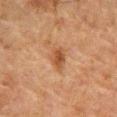Q: Is there a histopathology result?
A: imaged on a skin check; not biopsied
Q: What kind of image is this?
A: ~15 mm tile from a whole-body skin photo
Q: What are the patient's age and sex?
A: male, roughly 80 years of age
Q: Where on the body is the lesion?
A: the front of the torso